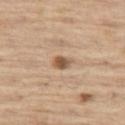biopsy status: imaged on a skin check; not biopsied
lesion size: ≈2.5 mm
automated lesion analysis: a border-irregularity rating of about 2/10, internal color variation of about 3 on a 0–10 scale, and radial color variation of about 1
image source: ~15 mm tile from a whole-body skin photo
location: the left thigh
patient: male, roughly 70 years of age